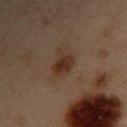A 15 mm close-up tile from a total-body photography series done for melanoma screening. Longest diameter approximately 4 mm. The total-body-photography lesion software estimated a lesion color around L≈25 a*≈13 b*≈21 in CIELAB, roughly 7 lightness units darker than nearby skin, and a normalized border contrast of about 8. The software also gave a classifier nevus-likeness of about 80/100 and lesion-presence confidence of about 100/100. Captured under cross-polarized illumination. The subject is a male aged 53–57. Located on the right upper arm.